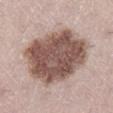Captured during whole-body skin photography for melanoma surveillance; the lesion was not biopsied. The lesion is located on the right lower leg. A female patient aged approximately 25. A 15 mm crop from a total-body photograph taken for skin-cancer surveillance.